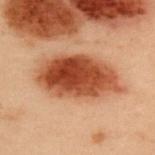{"biopsy_status": "not biopsied; imaged during a skin examination", "lesion_size": {"long_diameter_mm_approx": 9.0}, "lighting": "cross-polarized", "image": {"source": "total-body photography crop", "field_of_view_mm": 15}, "patient": {"sex": "male", "age_approx": 55}, "site": "upper back"}